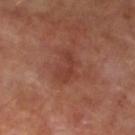Clinical impression: The lesion was photographed on a routine skin check and not biopsied; there is no pathology result. Clinical summary: Imaged with cross-polarized lighting. The subject is a male aged 68–72. Longest diameter approximately 3.5 mm. Located on the left lower leg. A 15 mm close-up extracted from a 3D total-body photography capture.